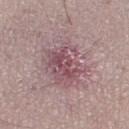Assessment: Recorded during total-body skin imaging; not selected for excision or biopsy. Background: On the right thigh. A male subject roughly 55 years of age. A 15 mm crop from a total-body photograph taken for skin-cancer surveillance. Captured under white-light illumination.